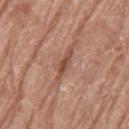| key | value |
|---|---|
| biopsy status | imaged on a skin check; not biopsied |
| subject | female, in their mid-70s |
| tile lighting | white-light |
| TBP lesion metrics | a lesion area of about 2.5 mm² and a shape-asymmetry score of about 0.35 (0 = symmetric) |
| body site | the left thigh |
| size | ≈3 mm |
| imaging modality | ~15 mm tile from a whole-body skin photo |